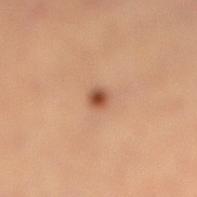Clinical impression: The lesion was tiled from a total-body skin photograph and was not biopsied. Clinical summary: A female subject, in their mid- to late 30s. A roughly 15 mm field-of-view crop from a total-body skin photograph. On the right lower leg. Automated image analysis of the tile measured a lesion area of about 2.5 mm², a shape eccentricity near 0.55, and a symmetry-axis asymmetry near 0.2. It also reported an average lesion color of about L≈50 a*≈23 b*≈33 (CIELAB). The software also gave a lesion-detection confidence of about 100/100. Approximately 2 mm at its widest. Imaged with cross-polarized lighting.